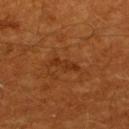biopsy status — total-body-photography surveillance lesion; no biopsy | subject — male, approximately 60 years of age | anatomic site — the upper back | acquisition — ~15 mm tile from a whole-body skin photo.